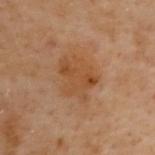The lesion was tiled from a total-body skin photograph and was not biopsied. A female subject, in their 60s. The lesion is located on the upper back. Cropped from a total-body skin-imaging series; the visible field is about 15 mm. Automated tile analysis of the lesion measured a lesion area of about 13 mm² and a symmetry-axis asymmetry near 0.25. The analysis additionally found a lesion color around L≈42 a*≈19 b*≈32 in CIELAB, about 6 CIELAB-L* units darker than the surrounding skin, and a lesion-to-skin contrast of about 6.5 (normalized; higher = more distinct). It also reported border irregularity of about 3 on a 0–10 scale and a within-lesion color-variation index near 3.5/10. The software also gave a classifier nevus-likeness of about 0/100 and a detector confidence of about 100 out of 100 that the crop contains a lesion. Captured under cross-polarized illumination.